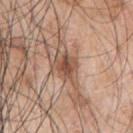Image and clinical context: A male subject aged 68 to 72. From the left arm. Captured under white-light illumination. A 15 mm close-up extracted from a 3D total-body photography capture. The lesion's longest dimension is about 3.5 mm.